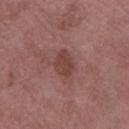Captured during whole-body skin photography for melanoma surveillance; the lesion was not biopsied.
The patient is a female roughly 70 years of age.
Approximately 3 mm at its widest.
A 15 mm close-up extracted from a 3D total-body photography capture.
On the left thigh.
Captured under white-light illumination.